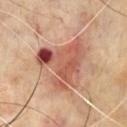biopsy status: imaged on a skin check; not biopsied
subject: male, aged 68 to 72
site: the chest
image source: ~15 mm tile from a whole-body skin photo
lighting: cross-polarized illumination
lesion size: about 6.5 mm